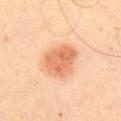Q: Is there a histopathology result?
A: imaged on a skin check; not biopsied
Q: Automated lesion metrics?
A: an area of roughly 12 mm²; a border-irregularity index near 2/10, a within-lesion color-variation index near 3.5/10, and radial color variation of about 1.5
Q: How large is the lesion?
A: ≈4.5 mm
Q: What kind of image is this?
A: ~15 mm tile from a whole-body skin photo
Q: What lighting was used for the tile?
A: cross-polarized illumination
Q: What are the patient's age and sex?
A: male, roughly 65 years of age
Q: What is the anatomic site?
A: the arm